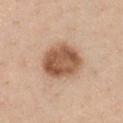| field | value |
|---|---|
| biopsy status | catalogued during a skin exam; not biopsied |
| lesion size | about 5 mm |
| location | the front of the torso |
| patient | female, approximately 40 years of age |
| image source | ~15 mm crop, total-body skin-cancer survey |
| illumination | white-light |
| automated lesion analysis | an area of roughly 17 mm² and an outline eccentricity of about 0.5 (0 = round, 1 = elongated); a lesion–skin lightness drop of about 15 and a normalized lesion–skin contrast near 10; a classifier nevus-likeness of about 50/100 and a lesion-detection confidence of about 100/100 |